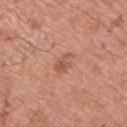Findings:
* follow-up — imaged on a skin check; not biopsied
* acquisition — 15 mm crop, total-body photography
* patient — male, in their mid- to late 60s
* anatomic site — the upper back